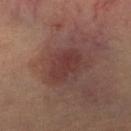Imaged during a routine full-body skin examination; the lesion was not biopsied and no histopathology is available.
Longest diameter approximately 4.5 mm.
A female patient aged around 65.
The lesion is on the right lower leg.
This image is a 15 mm lesion crop taken from a total-body photograph.
This is a cross-polarized tile.